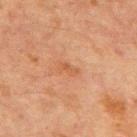The lesion was photographed on a routine skin check and not biopsied; there is no pathology result. From the front of the torso. This is a cross-polarized tile. A roughly 15 mm field-of-view crop from a total-body skin photograph. An algorithmic analysis of the crop reported border irregularity of about 3 on a 0–10 scale, a within-lesion color-variation index near 0/10, and peripheral color asymmetry of about 0. The patient is a male about 65 years old.